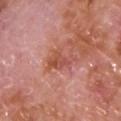No biopsy was performed on this lesion — it was imaged during a full skin examination and was not determined to be concerning.
A male subject, approximately 65 years of age.
The lesion is on the chest.
A 15 mm crop from a total-body photograph taken for skin-cancer surveillance.
Automated tile analysis of the lesion measured a footprint of about 7 mm² and a symmetry-axis asymmetry near 0.45. The analysis additionally found an average lesion color of about L≈52 a*≈29 b*≈31 (CIELAB), about 8 CIELAB-L* units darker than the surrounding skin, and a normalized lesion–skin contrast near 6.5. The software also gave a peripheral color-asymmetry measure near 1. It also reported a nevus-likeness score of about 0/100 and lesion-presence confidence of about 100/100.
The lesion's longest dimension is about 4.5 mm.